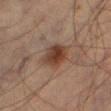Case summary:
* workup — total-body-photography surveillance lesion; no biopsy
* subject — male, aged around 65
* imaging modality — total-body-photography crop, ~15 mm field of view
* lighting — cross-polarized illumination
* lesion diameter — ~3.5 mm (longest diameter)
* site — the leg
* image-analysis metrics — two-axis asymmetry of about 0.2; an average lesion color of about L≈30 a*≈16 b*≈23 (CIELAB) and a normalized lesion–skin contrast near 10; an automated nevus-likeness rating near 95 out of 100